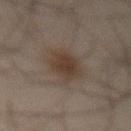The lesion-visualizer software estimated a nevus-likeness score of about 75/100 and lesion-presence confidence of about 100/100. Approximately 4 mm at its widest. A roughly 15 mm field-of-view crop from a total-body skin photograph. A male subject aged 43–47. The lesion is located on the abdomen.